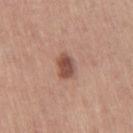This is a white-light tile. The subject is a female aged around 65. Approximately 3 mm at its widest. From the leg. The lesion-visualizer software estimated a border-irregularity index near 1.5/10 and a peripheral color-asymmetry measure near 1. It also reported a nevus-likeness score of about 95/100 and lesion-presence confidence of about 100/100. A 15 mm crop from a total-body photograph taken for skin-cancer surveillance.